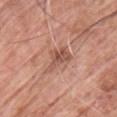Clinical impression:
The lesion was tiled from a total-body skin photograph and was not biopsied.
Background:
Longest diameter approximately 4 mm. This is a white-light tile. The lesion is on the chest. This image is a 15 mm lesion crop taken from a total-body photograph. A male patient in their 60s.